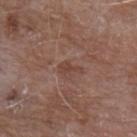Impression:
Recorded during total-body skin imaging; not selected for excision or biopsy.
Clinical summary:
The patient is a male about 80 years old. A 15 mm close-up extracted from a 3D total-body photography capture. Automated image analysis of the tile measured a border-irregularity rating of about 5.5/10, a within-lesion color-variation index near 0.5/10, and a peripheral color-asymmetry measure near 0.5. The lesion is located on the left forearm. Measured at roughly 2.5 mm in maximum diameter. Captured under white-light illumination.